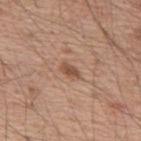Part of a total-body skin-imaging series; this lesion was reviewed on a skin check and was not flagged for biopsy. From the back. The recorded lesion diameter is about 2.5 mm. A lesion tile, about 15 mm wide, cut from a 3D total-body photograph. Imaged with white-light lighting. The subject is a male aged approximately 55.